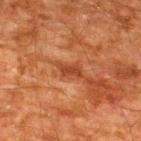No biopsy was performed on this lesion — it was imaged during a full skin examination and was not determined to be concerning. A lesion tile, about 15 mm wide, cut from a 3D total-body photograph. A male patient aged 58–62. On the back. About 3 mm across. Automated image analysis of the tile measured a border-irregularity index near 5/10, a color-variation rating of about 1/10, and radial color variation of about 0.5. It also reported an automated nevus-likeness rating near 0 out of 100.